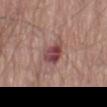Impression:
Recorded during total-body skin imaging; not selected for excision or biopsy.
Image and clinical context:
From the right thigh. The tile uses white-light illumination. The patient is a male in their 80s. This image is a 15 mm lesion crop taken from a total-body photograph. Longest diameter approximately 3.5 mm.